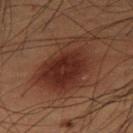{"site": "right thigh", "lighting": "cross-polarized", "patient": {"sex": "male", "age_approx": 50}, "image": {"source": "total-body photography crop", "field_of_view_mm": 15}, "lesion_size": {"long_diameter_mm_approx": 7.5}, "automated_metrics": {"cielab_L": 23, "cielab_a": 18, "cielab_b": 21, "vs_skin_darker_L": 8.0, "vs_skin_contrast_norm": 9.0, "nevus_likeness_0_100": 100, "lesion_detection_confidence_0_100": 100}}